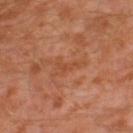body site: the left thigh | tile lighting: cross-polarized illumination | patient: male, aged 28–32 | image source: total-body-photography crop, ~15 mm field of view | lesion size: ~2.5 mm (longest diameter).